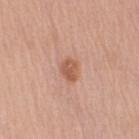follow-up: total-body-photography surveillance lesion; no biopsy
automated lesion analysis: an area of roughly 4.5 mm² and an outline eccentricity of about 0.65 (0 = round, 1 = elongated); an automated nevus-likeness rating near 80 out of 100 and lesion-presence confidence of about 100/100
patient: male, in their 60s
body site: the left upper arm
size: about 2.5 mm
imaging modality: 15 mm crop, total-body photography
illumination: white-light illumination